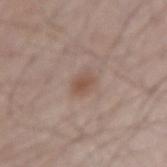notes: catalogued during a skin exam; not biopsied | location: the right forearm | subject: male, aged 63–67 | image: ~15 mm crop, total-body skin-cancer survey | size: ≈2.5 mm | automated lesion analysis: an area of roughly 5 mm², an outline eccentricity of about 0.6 (0 = round, 1 = elongated), and two-axis asymmetry of about 0.25; a mean CIELAB color near L≈52 a*≈17 b*≈26, roughly 8 lightness units darker than nearby skin, and a lesion-to-skin contrast of about 6.5 (normalized; higher = more distinct); radial color variation of about 0.5; an automated nevus-likeness rating near 70 out of 100 and a lesion-detection confidence of about 100/100.